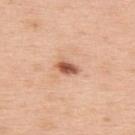Q: Is there a histopathology result?
A: total-body-photography surveillance lesion; no biopsy
Q: What lighting was used for the tile?
A: white-light illumination
Q: Who is the patient?
A: male, approximately 60 years of age
Q: What is the lesion's diameter?
A: ~2.5 mm (longest diameter)
Q: What did automated image analysis measure?
A: a footprint of about 3.5 mm², a shape eccentricity near 0.75, and a symmetry-axis asymmetry near 0.25; a border-irregularity rating of about 2/10, a color-variation rating of about 4/10, and a peripheral color-asymmetry measure near 1.5; an automated nevus-likeness rating near 95 out of 100 and lesion-presence confidence of about 100/100
Q: Lesion location?
A: the upper back
Q: What is the imaging modality?
A: total-body-photography crop, ~15 mm field of view